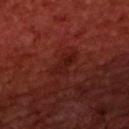Clinical impression:
Imaged during a routine full-body skin examination; the lesion was not biopsied and no histopathology is available.
Clinical summary:
About 3.5 mm across. A 15 mm crop from a total-body photograph taken for skin-cancer surveillance. Located on the upper back. A male subject aged 58 to 62. Imaged with cross-polarized lighting.